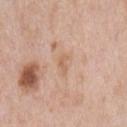Image and clinical context: On the chest. A 15 mm close-up extracted from a 3D total-body photography capture. A male patient, about 60 years old.